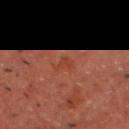Q: Is there a histopathology result?
A: no biopsy performed (imaged during a skin exam)
Q: How was this image acquired?
A: ~15 mm tile from a whole-body skin photo
Q: How large is the lesion?
A: ~2.5 mm (longest diameter)
Q: What are the patient's age and sex?
A: male, in their 60s
Q: Where on the body is the lesion?
A: the head or neck